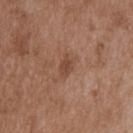Impression: The lesion was tiled from a total-body skin photograph and was not biopsied. Image and clinical context: The lesion is located on the back. A male subject aged around 50. A 15 mm close-up extracted from a 3D total-body photography capture. Captured under white-light illumination.